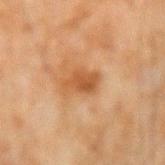Recorded during total-body skin imaging; not selected for excision or biopsy. The lesion is on the arm. Approximately 3.5 mm at its widest. A male subject aged 43 to 47. A lesion tile, about 15 mm wide, cut from a 3D total-body photograph.